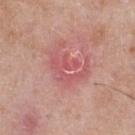Captured during whole-body skin photography for melanoma surveillance; the lesion was not biopsied. A male subject, about 50 years old. Cropped from a whole-body photographic skin survey; the tile spans about 15 mm. The lesion's longest dimension is about 4.5 mm. Located on the chest. The lesion-visualizer software estimated a mean CIELAB color near L≈56 a*≈32 b*≈23, a lesion–skin lightness drop of about 7, and a lesion-to-skin contrast of about 5 (normalized; higher = more distinct). The analysis additionally found a border-irregularity index near 8.5/10 and radial color variation of about 0.5. It also reported a nevus-likeness score of about 0/100 and a detector confidence of about 95 out of 100 that the crop contains a lesion. Captured under white-light illumination.